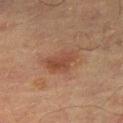{"lighting": "cross-polarized", "lesion_size": {"long_diameter_mm_approx": 4.5}, "patient": {"sex": "male", "age_approx": 65}, "site": "left thigh", "image": {"source": "total-body photography crop", "field_of_view_mm": 15}}